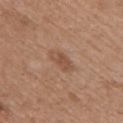This lesion was catalogued during total-body skin photography and was not selected for biopsy. A roughly 15 mm field-of-view crop from a total-body skin photograph. A female patient in their 60s. The lesion is located on the upper back.